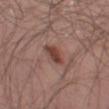Q: Was this lesion biopsied?
A: catalogued during a skin exam; not biopsied
Q: How was this image acquired?
A: ~15 mm tile from a whole-body skin photo
Q: Patient demographics?
A: male, in their mid-50s
Q: What is the anatomic site?
A: the left thigh
Q: What lighting was used for the tile?
A: white-light illumination
Q: What is the lesion's diameter?
A: ≈3.5 mm